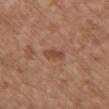notes = imaged on a skin check; not biopsied | body site = the chest | lighting = white-light | lesion size = about 3 mm | patient = female, about 75 years old | image source = ~15 mm crop, total-body skin-cancer survey.